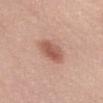The lesion was photographed on a routine skin check and not biopsied; there is no pathology result.
A 15 mm close-up extracted from a 3D total-body photography capture.
Located on the abdomen.
A female subject aged around 60.